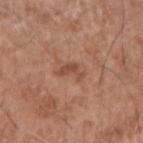Imaged during a routine full-body skin examination; the lesion was not biopsied and no histopathology is available. The subject is a male aged around 75. The lesion is located on the right upper arm. Measured at roughly 3 mm in maximum diameter. A roughly 15 mm field-of-view crop from a total-body skin photograph. Automated tile analysis of the lesion measured a footprint of about 3.5 mm², an outline eccentricity of about 0.8 (0 = round, 1 = elongated), and a shape-asymmetry score of about 0.6 (0 = symmetric). It also reported a lesion color around L≈49 a*≈23 b*≈30 in CIELAB, about 8 CIELAB-L* units darker than the surrounding skin, and a normalized lesion–skin contrast near 6.